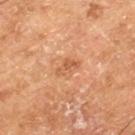This lesion was catalogued during total-body skin photography and was not selected for biopsy.